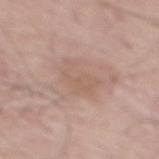<record>
  <biopsy_status>not biopsied; imaged during a skin examination</biopsy_status>
  <patient>
    <sex>male</sex>
    <age_approx>60</age_approx>
  </patient>
  <site>mid back</site>
  <image>
    <source>total-body photography crop</source>
    <field_of_view_mm>15</field_of_view_mm>
  </image>
</record>